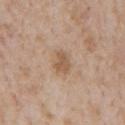Captured during whole-body skin photography for melanoma surveillance; the lesion was not biopsied.
The subject is a male in their mid-60s.
The lesion is located on the front of the torso.
Captured under white-light illumination.
Approximately 2.5 mm at its widest.
A 15 mm close-up extracted from a 3D total-body photography capture.